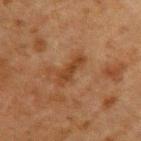Notes:
* notes: total-body-photography surveillance lesion; no biopsy
* acquisition: 15 mm crop, total-body photography
* patient: male, aged 48–52
* body site: the upper back